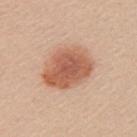Context:
Cropped from a total-body skin-imaging series; the visible field is about 15 mm. The subject is a female in their mid-20s. From the left upper arm. Captured under white-light illumination. The lesion-visualizer software estimated border irregularity of about 1.5 on a 0–10 scale, a within-lesion color-variation index near 4.5/10, and radial color variation of about 1.